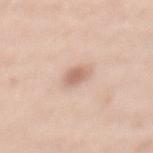follow-up = no biopsy performed (imaged during a skin exam)
lesion size = ≈3 mm
illumination = white-light illumination
patient = female, in their mid- to late 30s
anatomic site = the back
image = ~15 mm tile from a whole-body skin photo
image-analysis metrics = a mean CIELAB color near L≈66 a*≈19 b*≈28, about 11 CIELAB-L* units darker than the surrounding skin, and a normalized lesion–skin contrast near 6.5; a nevus-likeness score of about 90/100 and a detector confidence of about 100 out of 100 that the crop contains a lesion